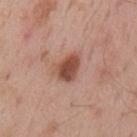Imaged during a routine full-body skin examination; the lesion was not biopsied and no histopathology is available.
The patient is a male aged approximately 55.
The lesion is on the mid back.
This is a white-light tile.
A roughly 15 mm field-of-view crop from a total-body skin photograph.
The lesion-visualizer software estimated an automated nevus-likeness rating near 95 out of 100 and a lesion-detection confidence of about 100/100.
The lesion's longest dimension is about 3.5 mm.